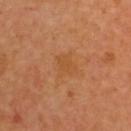Findings:
- follow-up · imaged on a skin check; not biopsied
- tile lighting · cross-polarized
- diameter · about 2.5 mm
- automated lesion analysis · a lesion area of about 5.5 mm², an eccentricity of roughly 0.6, and a shape-asymmetry score of about 0.35 (0 = symmetric); lesion-presence confidence of about 100/100
- subject · male, about 65 years old
- location · the upper back
- imaging modality · ~15 mm crop, total-body skin-cancer survey